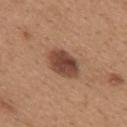{
  "biopsy_status": "not biopsied; imaged during a skin examination",
  "site": "upper back",
  "lighting": "white-light",
  "image": {
    "source": "total-body photography crop",
    "field_of_view_mm": 15
  },
  "automated_metrics": {
    "eccentricity": 0.75,
    "shape_asymmetry": 0.15,
    "cielab_L": 45,
    "cielab_a": 21,
    "cielab_b": 28,
    "vs_skin_darker_L": 14.0,
    "vs_skin_contrast_norm": 10.5,
    "lesion_detection_confidence_0_100": 100
  },
  "lesion_size": {
    "long_diameter_mm_approx": 4.5
  },
  "patient": {
    "sex": "female",
    "age_approx": 30
  }
}